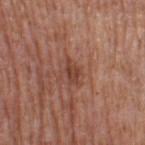Notes:
* follow-up: imaged on a skin check; not biopsied
* lighting: white-light illumination
* location: the left thigh
* diameter: ≈2.5 mm
* patient: female, in their mid- to late 60s
* acquisition: total-body-photography crop, ~15 mm field of view